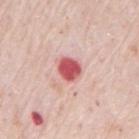  biopsy_status: not biopsied; imaged during a skin examination